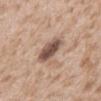Recorded during total-body skin imaging; not selected for excision or biopsy.
This is a white-light tile.
The subject is a male about 45 years old.
From the abdomen.
Longest diameter approximately 4 mm.
A 15 mm crop from a total-body photograph taken for skin-cancer surveillance.